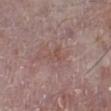Q: Was this lesion biopsied?
A: imaged on a skin check; not biopsied
Q: What is the lesion's diameter?
A: ~2.5 mm (longest diameter)
Q: What is the anatomic site?
A: the left lower leg
Q: What are the patient's age and sex?
A: male, aged 73 to 77
Q: What kind of image is this?
A: ~15 mm tile from a whole-body skin photo
Q: Illumination type?
A: white-light illumination